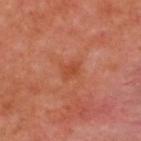| feature | finding |
|---|---|
| notes | catalogued during a skin exam; not biopsied |
| acquisition | ~15 mm crop, total-body skin-cancer survey |
| lesion size | ~2.5 mm (longest diameter) |
| patient | male, approximately 50 years of age |
| anatomic site | the upper back |
| tile lighting | cross-polarized |